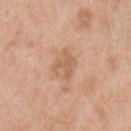Findings:
– lighting: white-light illumination
– image-analysis metrics: an area of roughly 5 mm² and an outline eccentricity of about 0.45 (0 = round, 1 = elongated); a mean CIELAB color near L≈60 a*≈21 b*≈34, roughly 8 lightness units darker than nearby skin, and a normalized lesion–skin contrast near 5.5; an automated nevus-likeness rating near 0 out of 100 and lesion-presence confidence of about 100/100
– image: ~15 mm tile from a whole-body skin photo
– subject: male, aged 48 to 52
– location: the right upper arm